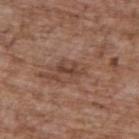The lesion was photographed on a routine skin check and not biopsied; there is no pathology result.
On the upper back.
A male patient, aged 68–72.
This is a white-light tile.
A region of skin cropped from a whole-body photographic capture, roughly 15 mm wide.
Longest diameter approximately 3.5 mm.